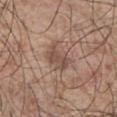Clinical impression:
Recorded during total-body skin imaging; not selected for excision or biopsy.
Image and clinical context:
A male patient, aged around 55. The lesion's longest dimension is about 3.5 mm. From the chest. This image is a 15 mm lesion crop taken from a total-body photograph. Automated image analysis of the tile measured a mean CIELAB color near L≈49 a*≈18 b*≈25 and about 9 CIELAB-L* units darker than the surrounding skin. It also reported a nevus-likeness score of about 10/100 and a lesion-detection confidence of about 100/100.